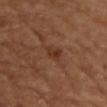Assessment:
Part of a total-body skin-imaging series; this lesion was reviewed on a skin check and was not flagged for biopsy.
Context:
The lesion is on the front of the torso. A region of skin cropped from a whole-body photographic capture, roughly 15 mm wide. Automated tile analysis of the lesion measured a footprint of about 3.5 mm², a shape eccentricity near 0.8, and a shape-asymmetry score of about 0.3 (0 = symmetric). And it measured a border-irregularity rating of about 3/10 and peripheral color asymmetry of about 1. About 3 mm across.